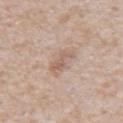No biopsy was performed on this lesion — it was imaged during a full skin examination and was not determined to be concerning.
A 15 mm close-up tile from a total-body photography series done for melanoma screening.
Located on the abdomen.
Captured under white-light illumination.
A male patient about 65 years old.